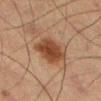Background:
A close-up tile cropped from a whole-body skin photograph, about 15 mm across. On the left thigh. A male patient in their mid- to late 60s.